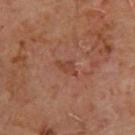workup: catalogued during a skin exam; not biopsied
subject: male, aged 63 to 67
imaging modality: 15 mm crop, total-body photography
diameter: about 3 mm
anatomic site: the upper back
image-analysis metrics: a footprint of about 3.5 mm², an outline eccentricity of about 0.8 (0 = round, 1 = elongated), and two-axis asymmetry of about 0.35; border irregularity of about 3.5 on a 0–10 scale, internal color variation of about 1.5 on a 0–10 scale, and a peripheral color-asymmetry measure near 0.5; a classifier nevus-likeness of about 0/100 and a detector confidence of about 100 out of 100 that the crop contains a lesion
lighting: cross-polarized illumination